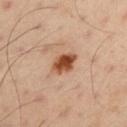notes: imaged on a skin check; not biopsied | image source: ~15 mm crop, total-body skin-cancer survey | subject: male, aged around 50 | lesion size: ≈3.5 mm | anatomic site: the left upper arm | automated lesion analysis: an average lesion color of about L≈50 a*≈24 b*≈34 (CIELAB), roughly 16 lightness units darker than nearby skin, and a lesion-to-skin contrast of about 11.5 (normalized; higher = more distinct); a classifier nevus-likeness of about 100/100 and a lesion-detection confidence of about 100/100.